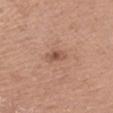Impression:
Part of a total-body skin-imaging series; this lesion was reviewed on a skin check and was not flagged for biopsy.
Image and clinical context:
Longest diameter approximately 2.5 mm. The tile uses white-light illumination. The lesion is located on the left upper arm. An algorithmic analysis of the crop reported an average lesion color of about L≈53 a*≈22 b*≈29 (CIELAB), about 9 CIELAB-L* units darker than the surrounding skin, and a normalized border contrast of about 6.5. And it measured border irregularity of about 2.5 on a 0–10 scale, a within-lesion color-variation index near 3.5/10, and a peripheral color-asymmetry measure near 1. It also reported an automated nevus-likeness rating near 15 out of 100. A 15 mm crop from a total-body photograph taken for skin-cancer surveillance. A female patient, aged approximately 40.